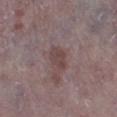Impression: Imaged during a routine full-body skin examination; the lesion was not biopsied and no histopathology is available. Acquisition and patient details: An algorithmic analysis of the crop reported roughly 8 lightness units darker than nearby skin and a normalized border contrast of about 6.5. A 15 mm close-up tile from a total-body photography series done for melanoma screening. The patient is a male aged 73 to 77. The lesion is on the left lower leg.